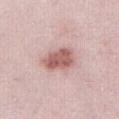Recorded during total-body skin imaging; not selected for excision or biopsy. A female patient roughly 30 years of age. The lesion-visualizer software estimated roughly 14 lightness units darker than nearby skin and a normalized lesion–skin contrast near 9. It also reported a border-irregularity index near 2.5/10 and a peripheral color-asymmetry measure near 1. The analysis additionally found a nevus-likeness score of about 75/100 and a detector confidence of about 100 out of 100 that the crop contains a lesion. About 4 mm across. Located on the abdomen. A 15 mm close-up tile from a total-body photography series done for melanoma screening.